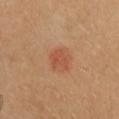Q: Is there a histopathology result?
A: no biopsy performed (imaged during a skin exam)
Q: What kind of image is this?
A: ~15 mm tile from a whole-body skin photo
Q: Patient demographics?
A: female, about 40 years old
Q: How was the tile lit?
A: cross-polarized illumination
Q: What is the anatomic site?
A: the head or neck